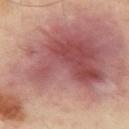Impression:
Recorded during total-body skin imaging; not selected for excision or biopsy.
Acquisition and patient details:
A male subject, about 50 years old. The lesion is on the left arm. About 19 mm across. Imaged with cross-polarized lighting. Cropped from a whole-body photographic skin survey; the tile spans about 15 mm.